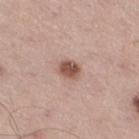{"biopsy_status": "not biopsied; imaged during a skin examination", "lesion_size": {"long_diameter_mm_approx": 2.5}, "patient": {"sex": "male", "age_approx": 55}, "lighting": "white-light", "image": {"source": "total-body photography crop", "field_of_view_mm": 15}, "automated_metrics": {"nevus_likeness_0_100": 95, "lesion_detection_confidence_0_100": 100}, "site": "right thigh"}